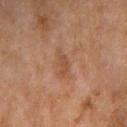This lesion was catalogued during total-body skin photography and was not selected for biopsy.
Captured under cross-polarized illumination.
Automated tile analysis of the lesion measured an area of roughly 4.5 mm² and a shape eccentricity near 0.85. And it measured a classifier nevus-likeness of about 0/100 and a detector confidence of about 100 out of 100 that the crop contains a lesion.
A close-up tile cropped from a whole-body skin photograph, about 15 mm across.
The subject is a female about 60 years old.
Located on the arm.
About 3 mm across.